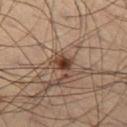The lesion was tiled from a total-body skin photograph and was not biopsied. Measured at roughly 2 mm in maximum diameter. From the left thigh. The total-body-photography lesion software estimated a footprint of about 3.5 mm². The analysis additionally found a mean CIELAB color near L≈38 a*≈19 b*≈27, roughly 13 lightness units darker than nearby skin, and a normalized lesion–skin contrast near 11. It also reported a border-irregularity rating of about 2.5/10 and a peripheral color-asymmetry measure near 2. A roughly 15 mm field-of-view crop from a total-body skin photograph. The subject is a male aged approximately 65. This is a cross-polarized tile.